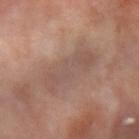<lesion>
<automated_metrics>
  <area_mm2_approx>17.0</area_mm2_approx>
  <eccentricity>0.9</eccentricity>
  <shape_asymmetry>0.2</shape_asymmetry>
  <cielab_L>51</cielab_L>
  <cielab_a>17</cielab_a>
  <cielab_b>23</cielab_b>
  <vs_skin_darker_L>6.0</vs_skin_darker_L>
  <vs_skin_contrast_norm>5.0</vs_skin_contrast_norm>
  <lesion_detection_confidence_0_100>95</lesion_detection_confidence_0_100>
</automated_metrics>
<patient>
  <sex>female</sex>
  <age_approx>60</age_approx>
</patient>
<site>arm</site>
<lighting>cross-polarized</lighting>
<lesion_size>
  <long_diameter_mm_approx>7.0</long_diameter_mm_approx>
</lesion_size>
<image>
  <source>total-body photography crop</source>
  <field_of_view_mm>15</field_of_view_mm>
</image>
</lesion>